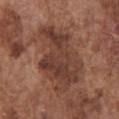No biopsy was performed on this lesion — it was imaged during a full skin examination and was not determined to be concerning. A male patient, aged 73–77. Captured under white-light illumination. Measured at roughly 9 mm in maximum diameter. The lesion is on the front of the torso. A close-up tile cropped from a whole-body skin photograph, about 15 mm across.